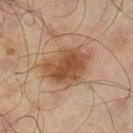Part of a total-body skin-imaging series; this lesion was reviewed on a skin check and was not flagged for biopsy. On the left lower leg. A male patient aged 63–67. A 15 mm close-up tile from a total-body photography series done for melanoma screening.